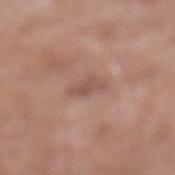  biopsy_status: not biopsied; imaged during a skin examination
  image:
    source: total-body photography crop
    field_of_view_mm: 15
  site: left lower leg
  patient:
    sex: male
    age_approx: 50